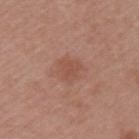{"biopsy_status": "not biopsied; imaged during a skin examination", "image": {"source": "total-body photography crop", "field_of_view_mm": 15}, "patient": {"sex": "female", "age_approx": 45}, "site": "arm"}